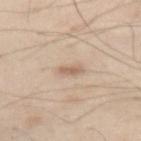Case summary:
* notes — no biopsy performed (imaged during a skin exam)
* body site — the abdomen
* subject — male, aged around 60
* image source — 15 mm crop, total-body photography